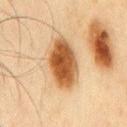Part of a total-body skin-imaging series; this lesion was reviewed on a skin check and was not flagged for biopsy. Longest diameter approximately 6.5 mm. Captured under cross-polarized illumination. The lesion is located on the chest. A male patient, aged 43–47. A roughly 15 mm field-of-view crop from a total-body skin photograph.